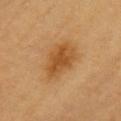biopsy status=total-body-photography surveillance lesion; no biopsy
location=the chest
patient=male, in their 60s
image=~15 mm crop, total-body skin-cancer survey